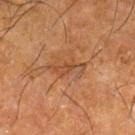biopsy status = imaged on a skin check; not biopsied
subject = male, aged 63–67
image source = total-body-photography crop, ~15 mm field of view
illumination = cross-polarized
location = the right lower leg
diameter = about 5 mm